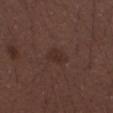This lesion was catalogued during total-body skin photography and was not selected for biopsy. Imaged with white-light lighting. A roughly 15 mm field-of-view crop from a total-body skin photograph. A male subject, approximately 30 years of age. Located on the left forearm. Longest diameter approximately 3 mm.